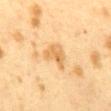Impression:
Part of a total-body skin-imaging series; this lesion was reviewed on a skin check and was not flagged for biopsy.
Context:
The total-body-photography lesion software estimated a lesion color around L≈57 a*≈17 b*≈39 in CIELAB, about 8 CIELAB-L* units darker than the surrounding skin, and a normalized lesion–skin contrast near 6.5. The software also gave a border-irregularity index near 4.5/10, internal color variation of about 2.5 on a 0–10 scale, and a peripheral color-asymmetry measure near 1. A lesion tile, about 15 mm wide, cut from a 3D total-body photograph. Imaged with cross-polarized lighting. About 3 mm across. A female subject, in their 40s. The lesion is on the mid back.